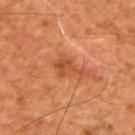This lesion was catalogued during total-body skin photography and was not selected for biopsy. A male patient approximately 65 years of age. From the upper back. Captured under cross-polarized illumination. About 4 mm across. A roughly 15 mm field-of-view crop from a total-body skin photograph. The lesion-visualizer software estimated internal color variation of about 2 on a 0–10 scale and a peripheral color-asymmetry measure near 0.5.